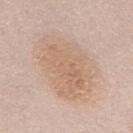<case>
<biopsy_status>not biopsied; imaged during a skin examination</biopsy_status>
<patient>
  <sex>female</sex>
  <age_approx>50</age_approx>
</patient>
<image>
  <source>total-body photography crop</source>
  <field_of_view_mm>15</field_of_view_mm>
</image>
<site>mid back</site>
<lighting>white-light</lighting>
<lesion_size>
  <long_diameter_mm_approx>9.5</long_diameter_mm_approx>
</lesion_size>
</case>